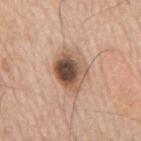Located on the back.
The patient is a male aged 63 to 67.
A lesion tile, about 15 mm wide, cut from a 3D total-body photograph.